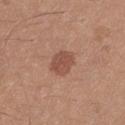- follow-up · no biopsy performed (imaged during a skin exam)
- site · the right upper arm
- patient · male, in their mid- to late 20s
- imaging modality · 15 mm crop, total-body photography
- lighting · white-light illumination
- lesion diameter · about 3 mm